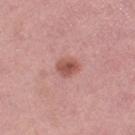This lesion was catalogued during total-body skin photography and was not selected for biopsy. Automated tile analysis of the lesion measured a lesion color around L≈53 a*≈26 b*≈26 in CIELAB, roughly 11 lightness units darker than nearby skin, and a normalized lesion–skin contrast near 8. A 15 mm crop from a total-body photograph taken for skin-cancer surveillance. A female patient aged 48 to 52. On the left thigh. Longest diameter approximately 2.5 mm. Captured under white-light illumination.